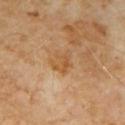Automated image analysis of the tile measured an area of roughly 5 mm², an eccentricity of roughly 0.6, and two-axis asymmetry of about 0.4. It also reported border irregularity of about 5.5 on a 0–10 scale, a within-lesion color-variation index near 2.5/10, and radial color variation of about 1. The software also gave an automated nevus-likeness rating near 0 out of 100 and a lesion-detection confidence of about 100/100. Located on the chest. About 3 mm across. A 15 mm crop from a total-body photograph taken for skin-cancer surveillance. Captured under cross-polarized illumination. A male patient, aged 58–62.